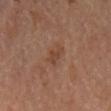The lesion was photographed on a routine skin check and not biopsied; there is no pathology result.